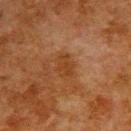Impression:
Captured during whole-body skin photography for melanoma surveillance; the lesion was not biopsied.
Background:
An algorithmic analysis of the crop reported internal color variation of about 1 on a 0–10 scale and a peripheral color-asymmetry measure near 0.5. Located on the upper back. A roughly 15 mm field-of-view crop from a total-body skin photograph. Longest diameter approximately 2.5 mm. Imaged with cross-polarized lighting. A male subject approximately 80 years of age.